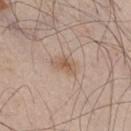Clinical impression: Imaged during a routine full-body skin examination; the lesion was not biopsied and no histopathology is available. Clinical summary: A male patient, about 45 years old. Imaged with white-light lighting. Automated tile analysis of the lesion measured an eccentricity of roughly 0.8 and two-axis asymmetry of about 0.4. The analysis additionally found a lesion color around L≈57 a*≈17 b*≈29 in CIELAB and about 9 CIELAB-L* units darker than the surrounding skin. And it measured a color-variation rating of about 2/10 and a peripheral color-asymmetry measure near 0.5. Approximately 3 mm at its widest. A region of skin cropped from a whole-body photographic capture, roughly 15 mm wide. On the leg.